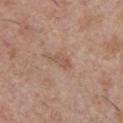| feature | finding |
|---|---|
| workup | imaged on a skin check; not biopsied |
| image source | total-body-photography crop, ~15 mm field of view |
| lesion size | about 2.5 mm |
| site | the chest |
| automated lesion analysis | a lesion color around L≈54 a*≈20 b*≈27 in CIELAB, about 7 CIELAB-L* units darker than the surrounding skin, and a normalized border contrast of about 5.5; lesion-presence confidence of about 100/100 |
| patient | male, approximately 30 years of age |